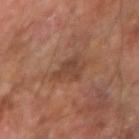The lesion was tiled from a total-body skin photograph and was not biopsied. An algorithmic analysis of the crop reported a lesion area of about 6 mm², an outline eccentricity of about 0.55 (0 = round, 1 = elongated), and a shape-asymmetry score of about 0.45 (0 = symmetric). This is a cross-polarized tile. The lesion is located on the right forearm. Cropped from a total-body skin-imaging series; the visible field is about 15 mm. The subject is a male aged 58 to 62. The recorded lesion diameter is about 3 mm.